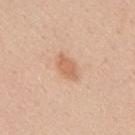{"biopsy_status": "not biopsied; imaged during a skin examination", "image": {"source": "total-body photography crop", "field_of_view_mm": 15}, "site": "mid back", "patient": {"sex": "male", "age_approx": 35}}